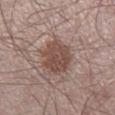Recorded during total-body skin imaging; not selected for excision or biopsy.